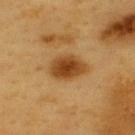| field | value |
|---|---|
| biopsy status | catalogued during a skin exam; not biopsied |
| patient | male, aged 58 to 62 |
| anatomic site | the upper back |
| illumination | cross-polarized |
| image | total-body-photography crop, ~15 mm field of view |